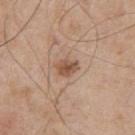<lesion>
  <biopsy_status>not biopsied; imaged during a skin examination</biopsy_status>
  <patient>
    <sex>male</sex>
    <age_approx>55</age_approx>
  </patient>
  <site>upper back</site>
  <lesion_size>
    <long_diameter_mm_approx>2.5</long_diameter_mm_approx>
  </lesion_size>
  <image>
    <source>total-body photography crop</source>
    <field_of_view_mm>15</field_of_view_mm>
  </image>
</lesion>